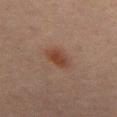Image and clinical context:
A 15 mm close-up tile from a total-body photography series done for melanoma screening. The lesion is on the mid back. Captured under cross-polarized illumination. About 3 mm across. The patient is a male aged approximately 70. The total-body-photography lesion software estimated an area of roughly 5.5 mm², a shape eccentricity near 0.7, and a symmetry-axis asymmetry near 0.2.